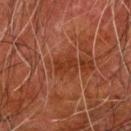Background:
The lesion-visualizer software estimated a footprint of about 3.5 mm², a shape eccentricity near 0.85, and a shape-asymmetry score of about 0.35 (0 = symmetric). The software also gave border irregularity of about 4 on a 0–10 scale and peripheral color asymmetry of about 0. The software also gave a nevus-likeness score of about 0/100 and a lesion-detection confidence of about 100/100. A male subject, approximately 80 years of age. The tile uses cross-polarized illumination. A roughly 15 mm field-of-view crop from a total-body skin photograph. From the arm. Approximately 2.5 mm at its widest.